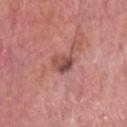Clinical impression: Part of a total-body skin-imaging series; this lesion was reviewed on a skin check and was not flagged for biopsy. Context: A close-up tile cropped from a whole-body skin photograph, about 15 mm across. From the head or neck. Imaged with white-light lighting. A male subject aged approximately 75. The recorded lesion diameter is about 3 mm.